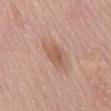Imaged during a routine full-body skin examination; the lesion was not biopsied and no histopathology is available.
A 15 mm crop from a total-body photograph taken for skin-cancer surveillance.
Imaged with white-light lighting.
A female patient, about 65 years old.
On the mid back.